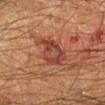| feature | finding |
|---|---|
| biopsy status | imaged on a skin check; not biopsied |
| illumination | cross-polarized |
| image source | 15 mm crop, total-body photography |
| patient | male, aged 63 to 67 |
| lesion size | ≈4 mm |
| automated lesion analysis | border irregularity of about 3 on a 0–10 scale and internal color variation of about 5 on a 0–10 scale; lesion-presence confidence of about 100/100 |
| anatomic site | the left lower leg |